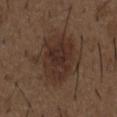Q: Automated lesion metrics?
A: an average lesion color of about L≈30 a*≈16 b*≈22 (CIELAB), a lesion–skin lightness drop of about 8, and a normalized lesion–skin contrast near 8.5; internal color variation of about 4 on a 0–10 scale and radial color variation of about 1; a classifier nevus-likeness of about 35/100 and lesion-presence confidence of about 100/100
Q: How large is the lesion?
A: about 6.5 mm
Q: Lesion location?
A: the chest
Q: Illumination type?
A: white-light
Q: What kind of image is this?
A: total-body-photography crop, ~15 mm field of view
Q: Patient demographics?
A: male, approximately 50 years of age